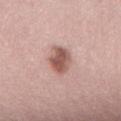Clinical impression:
The lesion was photographed on a routine skin check and not biopsied; there is no pathology result.
Acquisition and patient details:
The patient is a female aged 48 to 52. Measured at roughly 3.5 mm in maximum diameter. This image is a 15 mm lesion crop taken from a total-body photograph. The tile uses white-light illumination. Automated tile analysis of the lesion measured a mean CIELAB color near L≈55 a*≈22 b*≈25, about 14 CIELAB-L* units darker than the surrounding skin, and a normalized lesion–skin contrast near 9.5. Located on the back.